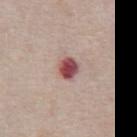{"biopsy_status": "not biopsied; imaged during a skin examination", "patient": {"sex": "male", "age_approx": 75}, "image": {"source": "total-body photography crop", "field_of_view_mm": 15}, "site": "front of the torso", "lighting": "white-light"}